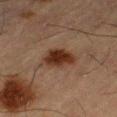biopsy status = no biopsy performed (imaged during a skin exam)
tile lighting = cross-polarized
imaging modality = total-body-photography crop, ~15 mm field of view
patient = male, aged 63 to 67
body site = the right thigh